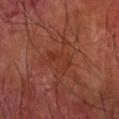Clinical impression: Part of a total-body skin-imaging series; this lesion was reviewed on a skin check and was not flagged for biopsy. Acquisition and patient details: The lesion is on the right forearm. A male patient aged 68 to 72. This is a cross-polarized tile. Automated tile analysis of the lesion measured an area of roughly 3.5 mm² and a shape-asymmetry score of about 0.55 (0 = symmetric). The analysis additionally found an average lesion color of about L≈34 a*≈28 b*≈31 (CIELAB), a lesion–skin lightness drop of about 5, and a lesion-to-skin contrast of about 5.5 (normalized; higher = more distinct). It also reported a border-irregularity rating of about 7/10, a within-lesion color-variation index near 0/10, and radial color variation of about 0. And it measured an automated nevus-likeness rating near 0 out of 100 and a detector confidence of about 100 out of 100 that the crop contains a lesion. A close-up tile cropped from a whole-body skin photograph, about 15 mm across.